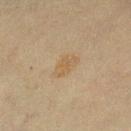biopsy_status: not biopsied; imaged during a skin examination
image:
  source: total-body photography crop
  field_of_view_mm: 15
site: leg
lesion_size:
  long_diameter_mm_approx: 3.5
patient:
  sex: female
  age_approx: 55
lighting: cross-polarized